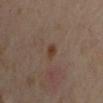biopsy status: no biopsy performed (imaged during a skin exam) | imaging modality: ~15 mm tile from a whole-body skin photo | image-analysis metrics: an eccentricity of roughly 0.7 and a shape-asymmetry score of about 0.3 (0 = symmetric); a mean CIELAB color near L≈36 a*≈16 b*≈26, a lesion–skin lightness drop of about 8, and a normalized border contrast of about 8.5; border irregularity of about 2 on a 0–10 scale, a color-variation rating of about 1/10, and radial color variation of about 0.5; a classifier nevus-likeness of about 95/100 | location: the right upper arm | subject: male, aged approximately 45.